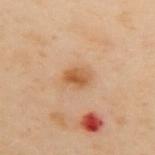illumination = cross-polarized illumination | imaging modality = 15 mm crop, total-body photography | patient = female, approximately 60 years of age | site = the upper back | automated metrics = roughly 9 lightness units darker than nearby skin and a normalized border contrast of about 8; border irregularity of about 1.5 on a 0–10 scale, a within-lesion color-variation index near 4/10, and radial color variation of about 1.5; a nevus-likeness score of about 95/100 and a detector confidence of about 100 out of 100 that the crop contains a lesion | lesion diameter = about 3 mm.